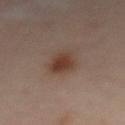pathology: an atypical melanocytic neoplasm — a borderline lesion of uncertain malignant potential.